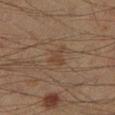imaging modality = 15 mm crop, total-body photography
subject = male, approximately 40 years of age
location = the leg
tile lighting = cross-polarized illumination
lesion diameter = about 3 mm